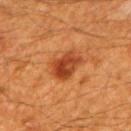{
  "biopsy_status": "not biopsied; imaged during a skin examination",
  "patient": {
    "sex": "male",
    "age_approx": 60
  },
  "lesion_size": {
    "long_diameter_mm_approx": 4.0
  },
  "lighting": "cross-polarized",
  "image": {
    "source": "total-body photography crop",
    "field_of_view_mm": 15
  },
  "site": "mid back"
}